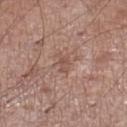This lesion was catalogued during total-body skin photography and was not selected for biopsy.
The patient is a male approximately 55 years of age.
Measured at roughly 2.5 mm in maximum diameter.
A close-up tile cropped from a whole-body skin photograph, about 15 mm across.
On the left lower leg.